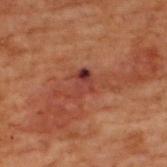Captured during whole-body skin photography for melanoma surveillance; the lesion was not biopsied. Automated image analysis of the tile measured a border-irregularity index near 4/10, a color-variation rating of about 6/10, and peripheral color asymmetry of about 2. The analysis additionally found an automated nevus-likeness rating near 0 out of 100 and a lesion-detection confidence of about 100/100. Located on the upper back. A male patient in their 70s. Measured at roughly 3 mm in maximum diameter. A lesion tile, about 15 mm wide, cut from a 3D total-body photograph. The tile uses cross-polarized illumination.